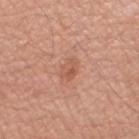<case>
<biopsy_status>not biopsied; imaged during a skin examination</biopsy_status>
<site>arm</site>
<patient>
  <sex>male</sex>
  <age_approx>70</age_approx>
</patient>
<image>
  <source>total-body photography crop</source>
  <field_of_view_mm>15</field_of_view_mm>
</image>
<lighting>white-light</lighting>
</case>